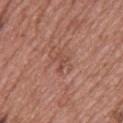| field | value |
|---|---|
| notes | total-body-photography surveillance lesion; no biopsy |
| site | the back |
| acquisition | ~15 mm crop, total-body skin-cancer survey |
| lesion size | ≈2.5 mm |
| patient | female, about 60 years old |
| lighting | white-light |